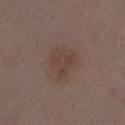Q: Automated lesion metrics?
A: a footprint of about 9.5 mm², an eccentricity of roughly 0.5, and a symmetry-axis asymmetry near 0.35; a color-variation rating of about 2/10; a classifier nevus-likeness of about 15/100 and a lesion-detection confidence of about 100/100
Q: How large is the lesion?
A: ~4 mm (longest diameter)
Q: How was this image acquired?
A: ~15 mm tile from a whole-body skin photo
Q: Patient demographics?
A: female, aged around 30
Q: What is the anatomic site?
A: the mid back